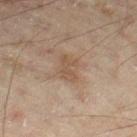The lesion was tiled from a total-body skin photograph and was not biopsied. This is a cross-polarized tile. The lesion is located on the right lower leg. A roughly 15 mm field-of-view crop from a total-body skin photograph. A male patient aged 43–47. The recorded lesion diameter is about 3.5 mm.